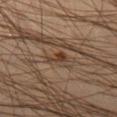<tbp_lesion>
<site>left thigh</site>
<lesion_size>
  <long_diameter_mm_approx>3.0</long_diameter_mm_approx>
</lesion_size>
<patient>
  <sex>male</sex>
  <age_approx>45</age_approx>
</patient>
<image>
  <source>total-body photography crop</source>
  <field_of_view_mm>15</field_of_view_mm>
</image>
<lighting>cross-polarized</lighting>
</tbp_lesion>